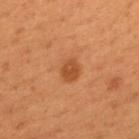Q: Is there a histopathology result?
A: total-body-photography surveillance lesion; no biopsy
Q: What did automated image analysis measure?
A: about 6 CIELAB-L* units darker than the surrounding skin and a normalized lesion–skin contrast near 5.5
Q: How large is the lesion?
A: ~3.5 mm (longest diameter)
Q: Who is the patient?
A: male, aged 48 to 52
Q: Lesion location?
A: the mid back
Q: What lighting was used for the tile?
A: cross-polarized
Q: What is the imaging modality?
A: ~15 mm tile from a whole-body skin photo